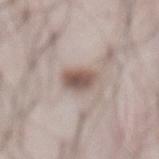Q: Is there a histopathology result?
A: total-body-photography surveillance lesion; no biopsy
Q: Where on the body is the lesion?
A: the abdomen
Q: Patient demographics?
A: male, in their 70s
Q: Illumination type?
A: white-light
Q: What kind of image is this?
A: total-body-photography crop, ~15 mm field of view